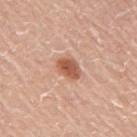Imaged during a routine full-body skin examination; the lesion was not biopsied and no histopathology is available.
A region of skin cropped from a whole-body photographic capture, roughly 15 mm wide.
Imaged with white-light lighting.
The lesion is on the arm.
Automated image analysis of the tile measured a mean CIELAB color near L≈56 a*≈24 b*≈33 and a lesion-to-skin contrast of about 9 (normalized; higher = more distinct). And it measured a border-irregularity rating of about 2/10, internal color variation of about 3.5 on a 0–10 scale, and a peripheral color-asymmetry measure near 1.
The patient is a male roughly 60 years of age.